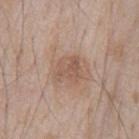{"automated_metrics": {"border_irregularity_0_10": 3.0, "color_variation_0_10": 3.0, "peripheral_color_asymmetry": 1.0}, "patient": {"sex": "male", "age_approx": 65}, "image": {"source": "total-body photography crop", "field_of_view_mm": 15}, "lighting": "white-light", "site": "front of the torso", "lesion_size": {"long_diameter_mm_approx": 3.5}}